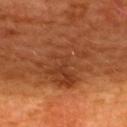Recorded during total-body skin imaging; not selected for excision or biopsy.
A 15 mm close-up extracted from a 3D total-body photography capture.
On the upper back.
A female subject, in their mid-40s.